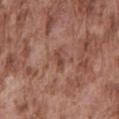Part of a total-body skin-imaging series; this lesion was reviewed on a skin check and was not flagged for biopsy.
The subject is a male aged 73–77.
The lesion is located on the mid back.
A 15 mm close-up extracted from a 3D total-body photography capture.